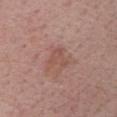Q: Was this lesion biopsied?
A: total-body-photography surveillance lesion; no biopsy
Q: Where on the body is the lesion?
A: the chest
Q: How was this image acquired?
A: ~15 mm tile from a whole-body skin photo
Q: What are the patient's age and sex?
A: female, aged 48–52
Q: Lesion size?
A: ≈3 mm